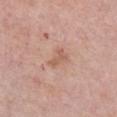Imaged during a routine full-body skin examination; the lesion was not biopsied and no histopathology is available. An algorithmic analysis of the crop reported a mean CIELAB color near L≈59 a*≈22 b*≈29, a lesion–skin lightness drop of about 8, and a normalized border contrast of about 5.5. It also reported a classifier nevus-likeness of about 0/100 and lesion-presence confidence of about 100/100. A close-up tile cropped from a whole-body skin photograph, about 15 mm across. Located on the front of the torso. A female subject, approximately 65 years of age. Measured at roughly 3 mm in maximum diameter.